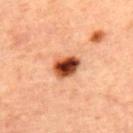No biopsy was performed on this lesion — it was imaged during a full skin examination and was not determined to be concerning.
A roughly 15 mm field-of-view crop from a total-body skin photograph.
Approximately 3.5 mm at its widest.
A female patient approximately 45 years of age.
From the upper back.
This is a cross-polarized tile.